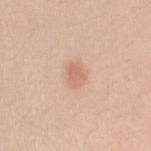Assessment: Captured during whole-body skin photography for melanoma surveillance; the lesion was not biopsied. Background: A lesion tile, about 15 mm wide, cut from a 3D total-body photograph. Automated tile analysis of the lesion measured border irregularity of about 2.5 on a 0–10 scale and a color-variation rating of about 2/10. The software also gave a classifier nevus-likeness of about 80/100. On the arm. A female patient in their mid-20s. Captured under white-light illumination. The lesion's longest dimension is about 2.5 mm.